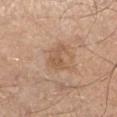Impression:
Imaged during a routine full-body skin examination; the lesion was not biopsied and no histopathology is available.
Acquisition and patient details:
The lesion is on the left lower leg. A male subject approximately 55 years of age. A 15 mm close-up extracted from a 3D total-body photography capture.